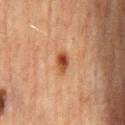The lesion was tiled from a total-body skin photograph and was not biopsied.
The recorded lesion diameter is about 2.5 mm.
The lesion-visualizer software estimated a mean CIELAB color near L≈40 a*≈22 b*≈31 and a lesion-to-skin contrast of about 9.5 (normalized; higher = more distinct). And it measured a border-irregularity index near 2/10, a within-lesion color-variation index near 4/10, and a peripheral color-asymmetry measure near 1. It also reported a lesion-detection confidence of about 100/100.
The patient is a male aged approximately 65.
A 15 mm close-up tile from a total-body photography series done for melanoma screening.
Located on the abdomen.
The tile uses cross-polarized illumination.